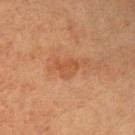<case>
<biopsy_status>not biopsied; imaged during a skin examination</biopsy_status>
<image>
  <source>total-body photography crop</source>
  <field_of_view_mm>15</field_of_view_mm>
</image>
<lesion_size>
  <long_diameter_mm_approx>3.0</long_diameter_mm_approx>
</lesion_size>
<automated_metrics>
  <peripheral_color_asymmetry>0.5</peripheral_color_asymmetry>
  <lesion_detection_confidence_0_100>100</lesion_detection_confidence_0_100>
</automated_metrics>
<patient>
  <sex>male</sex>
  <age_approx>60</age_approx>
</patient>
<lighting>cross-polarized</lighting>
<site>upper back</site>
</case>